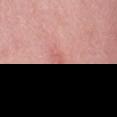<lesion>
<biopsy_status>not biopsied; imaged during a skin examination</biopsy_status>
<lighting>white-light</lighting>
<image>
  <source>total-body photography crop</source>
  <field_of_view_mm>15</field_of_view_mm>
</image>
<site>mid back</site>
<patient>
  <sex>female</sex>
  <age_approx>60</age_approx>
</patient>
<automated_metrics>
  <cielab_L>60</cielab_L>
  <cielab_a>29</cielab_a>
  <cielab_b>26</cielab_b>
  <vs_skin_darker_L>6.0</vs_skin_darker_L>
  <vs_skin_contrast_norm>4.0</vs_skin_contrast_norm>
  <nevus_likeness_0_100>0</nevus_likeness_0_100>
  <lesion_detection_confidence_0_100>70</lesion_detection_confidence_0_100>
</automated_metrics>
</lesion>